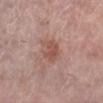Clinical summary: The subject is a female about 65 years old. Automated tile analysis of the lesion measured an area of roughly 6 mm², an eccentricity of roughly 0.55, and a symmetry-axis asymmetry near 0.25. The software also gave a border-irregularity index near 3/10, internal color variation of about 2 on a 0–10 scale, and a peripheral color-asymmetry measure near 0.5. Imaged with white-light lighting. On the left lower leg. The recorded lesion diameter is about 3 mm. Cropped from a whole-body photographic skin survey; the tile spans about 15 mm.